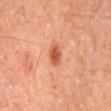A lesion tile, about 15 mm wide, cut from a 3D total-body photograph. Longest diameter approximately 2.5 mm. The subject is a male roughly 60 years of age. Captured under cross-polarized illumination. An algorithmic analysis of the crop reported an outline eccentricity of about 0.75 (0 = round, 1 = elongated) and a shape-asymmetry score of about 0.2 (0 = symmetric). The software also gave a lesion color around L≈42 a*≈24 b*≈30 in CIELAB and a lesion–skin lightness drop of about 10. The software also gave a classifier nevus-likeness of about 95/100 and lesion-presence confidence of about 100/100. From the mid back.